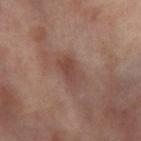Recorded during total-body skin imaging; not selected for excision or biopsy.
A female patient roughly 55 years of age.
From the right thigh.
A 15 mm close-up extracted from a 3D total-body photography capture.